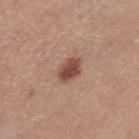Imaged with white-light lighting.
Located on the left thigh.
A 15 mm close-up tile from a total-body photography series done for melanoma screening.
A female patient aged 38–42.